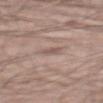biopsy status: imaged on a skin check; not biopsied
anatomic site: the back
acquisition: ~15 mm crop, total-body skin-cancer survey
subject: male, aged 53 to 57